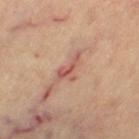Q: Was this lesion biopsied?
A: total-body-photography surveillance lesion; no biopsy
Q: What are the patient's age and sex?
A: female, roughly 65 years of age
Q: What kind of image is this?
A: 15 mm crop, total-body photography
Q: Where on the body is the lesion?
A: the left thigh